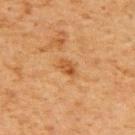<record>
  <biopsy_status>not biopsied; imaged during a skin examination</biopsy_status>
  <site>back</site>
  <image>
    <source>total-body photography crop</source>
    <field_of_view_mm>15</field_of_view_mm>
  </image>
  <patient>
    <sex>male</sex>
    <age_approx>60</age_approx>
  </patient>
</record>